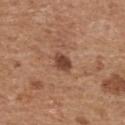follow-up: total-body-photography surveillance lesion; no biopsy
image: 15 mm crop, total-body photography
location: the upper back
lesion diameter: ~2.5 mm (longest diameter)
subject: male, aged 68–72
tile lighting: white-light
automated lesion analysis: an area of roughly 4 mm², a shape eccentricity near 0.75, and two-axis asymmetry of about 0.2